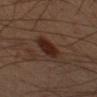Q: Was a biopsy performed?
A: total-body-photography surveillance lesion; no biopsy
Q: Lesion location?
A: the left forearm
Q: What is the imaging modality?
A: ~15 mm tile from a whole-body skin photo
Q: Who is the patient?
A: male, approximately 50 years of age